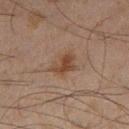Impression: The lesion was tiled from a total-body skin photograph and was not biopsied. Background: Automated tile analysis of the lesion measured a mean CIELAB color near L≈36 a*≈15 b*≈24, a lesion–skin lightness drop of about 7, and a normalized lesion–skin contrast near 7. The software also gave a border-irregularity index near 3/10, a within-lesion color-variation index near 4/10, and radial color variation of about 1. The analysis additionally found an automated nevus-likeness rating near 65 out of 100 and a lesion-detection confidence of about 100/100. On the left lower leg. A region of skin cropped from a whole-body photographic capture, roughly 15 mm wide. A male subject, aged approximately 45.